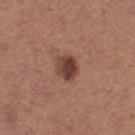follow-up: total-body-photography surveillance lesion; no biopsy
size: ≈2.5 mm
subject: female, aged 28–32
location: the left thigh
illumination: white-light illumination
acquisition: total-body-photography crop, ~15 mm field of view
image-analysis metrics: a lesion area of about 6 mm² and a symmetry-axis asymmetry near 0.15